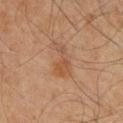size: ≈4.5 mm
image source: total-body-photography crop, ~15 mm field of view
illumination: cross-polarized illumination
automated lesion analysis: a footprint of about 7.5 mm², an eccentricity of roughly 0.9, and a symmetry-axis asymmetry near 0.5; border irregularity of about 6 on a 0–10 scale, internal color variation of about 3 on a 0–10 scale, and peripheral color asymmetry of about 1; a classifier nevus-likeness of about 0/100 and lesion-presence confidence of about 100/100
patient: male, aged 58 to 62
anatomic site: the chest